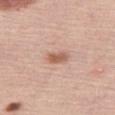Case summary:
– biopsy status: catalogued during a skin exam; not biopsied
– imaging modality: ~15 mm tile from a whole-body skin photo
– automated lesion analysis: a lesion area of about 4 mm² and a symmetry-axis asymmetry near 0.3; border irregularity of about 2.5 on a 0–10 scale, a color-variation rating of about 3/10, and a peripheral color-asymmetry measure near 1; lesion-presence confidence of about 100/100
– tile lighting: white-light
– lesion size: ≈2.5 mm
– body site: the right thigh
– subject: female, in their mid- to late 60s